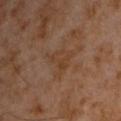Q: Is there a histopathology result?
A: no biopsy performed (imaged during a skin exam)
Q: How was this image acquired?
A: ~15 mm crop, total-body skin-cancer survey
Q: Lesion location?
A: the chest
Q: Who is the patient?
A: male, aged around 60